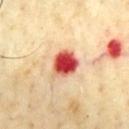notes: total-body-photography surveillance lesion; no biopsy